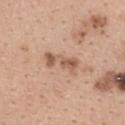* biopsy status: catalogued during a skin exam; not biopsied
* patient: female, aged approximately 40
* location: the upper back
* imaging modality: ~15 mm tile from a whole-body skin photo